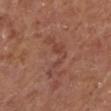<record>
<biopsy_status>not biopsied; imaged during a skin examination</biopsy_status>
<lighting>white-light</lighting>
<lesion_size>
  <long_diameter_mm_approx>5.0</long_diameter_mm_approx>
</lesion_size>
<patient>
  <sex>male</sex>
  <age_approx>75</age_approx>
</patient>
<image>
  <source>total-body photography crop</source>
  <field_of_view_mm>15</field_of_view_mm>
</image>
<site>left lower leg</site>
</record>